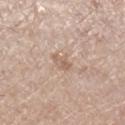The lesion was tiled from a total-body skin photograph and was not biopsied. Automated tile analysis of the lesion measured an area of roughly 3 mm², a shape eccentricity near 0.8, and a shape-asymmetry score of about 0.25 (0 = symmetric). The software also gave a mean CIELAB color near L≈60 a*≈17 b*≈28 and about 8 CIELAB-L* units darker than the surrounding skin. The software also gave a border-irregularity rating of about 2.5/10, internal color variation of about 2 on a 0–10 scale, and peripheral color asymmetry of about 0.5. The software also gave an automated nevus-likeness rating near 0 out of 100 and lesion-presence confidence of about 100/100. On the right lower leg. The patient is a female aged around 55. A 15 mm crop from a total-body photograph taken for skin-cancer surveillance. The tile uses white-light illumination.